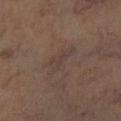Impression:
This lesion was catalogued during total-body skin photography and was not selected for biopsy.
Clinical summary:
On the right lower leg. This image is a 15 mm lesion crop taken from a total-body photograph. Captured under cross-polarized illumination. Approximately 4 mm at its widest. A subject aged 63 to 67.